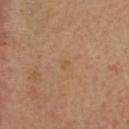The lesion was photographed on a routine skin check and not biopsied; there is no pathology result.